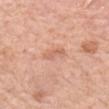The lesion was photographed on a routine skin check and not biopsied; there is no pathology result.
The tile uses white-light illumination.
The lesion is on the left forearm.
The recorded lesion diameter is about 3 mm.
A female subject, aged around 55.
A 15 mm close-up tile from a total-body photography series done for melanoma screening.